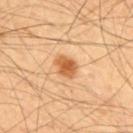  site: upper back
  patient:
    sex: male
    age_approx: 55
  image:
    source: total-body photography crop
    field_of_view_mm: 15